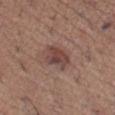Clinical impression: The lesion was tiled from a total-body skin photograph and was not biopsied. Image and clinical context: A lesion tile, about 15 mm wide, cut from a 3D total-body photograph. Approximately 3.5 mm at its widest. Imaged with white-light lighting. The lesion is on the left thigh. The subject is a female about 35 years old.